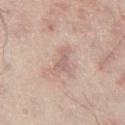  biopsy_status: not biopsied; imaged during a skin examination
  image:
    source: total-body photography crop
    field_of_view_mm: 15
  automated_metrics:
    area_mm2_approx: 3.5
    eccentricity: 0.85
    shape_asymmetry: 0.55
  lighting: white-light
  site: right thigh
  patient:
    sex: male
    age_approx: 65